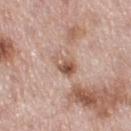Impression: The lesion was photographed on a routine skin check and not biopsied; there is no pathology result.